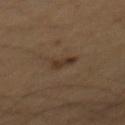* notes — catalogued during a skin exam; not biopsied